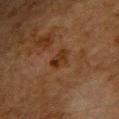biopsy status = catalogued during a skin exam; not biopsied
diameter = ~2.5 mm (longest diameter)
subject = female, approximately 55 years of age
anatomic site = the right upper arm
image source = ~15 mm crop, total-body skin-cancer survey